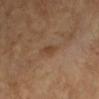Clinical impression: No biopsy was performed on this lesion — it was imaged during a full skin examination and was not determined to be concerning. Context: Located on the left forearm. A region of skin cropped from a whole-body photographic capture, roughly 15 mm wide. The patient is aged 58 to 62.